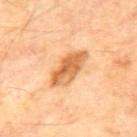Clinical summary:
The total-body-photography lesion software estimated a normalized lesion–skin contrast near 9. It also reported border irregularity of about 2.5 on a 0–10 scale and internal color variation of about 6 on a 0–10 scale. The subject is a male aged 68 to 72. A roughly 15 mm field-of-view crop from a total-body skin photograph. On the back. Captured under cross-polarized illumination.